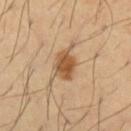notes: catalogued during a skin exam; not biopsied | location: the left upper arm | subject: male, approximately 40 years of age | acquisition: ~15 mm crop, total-body skin-cancer survey.